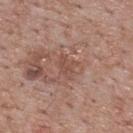biopsy_status: not biopsied; imaged during a skin examination
lighting: white-light
lesion_size:
  long_diameter_mm_approx: 2.5
patient:
  sex: male
  age_approx: 70
automated_metrics:
  cielab_L: 49
  cielab_a: 21
  cielab_b: 26
  vs_skin_contrast_norm: 5.5
  border_irregularity_0_10: 3.5
  color_variation_0_10: 0.5
  peripheral_color_asymmetry: 0.0
  nevus_likeness_0_100: 0
image:
  source: total-body photography crop
  field_of_view_mm: 15
site: upper back